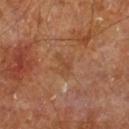Impression: Captured during whole-body skin photography for melanoma surveillance; the lesion was not biopsied. Background: Automated tile analysis of the lesion measured a border-irregularity rating of about 5.5/10, internal color variation of about 0 on a 0–10 scale, and peripheral color asymmetry of about 0. The software also gave an automated nevus-likeness rating near 0 out of 100 and a lesion-detection confidence of about 100/100. The lesion is on the right leg. Longest diameter approximately 2.5 mm. Cropped from a total-body skin-imaging series; the visible field is about 15 mm. The subject is a male aged approximately 60.